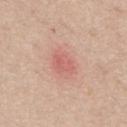Notes:
• workup · total-body-photography surveillance lesion; no biopsy
• tile lighting · white-light
• diameter · ~3.5 mm (longest diameter)
• patient · male, aged approximately 60
• image source · ~15 mm tile from a whole-body skin photo
• location · the mid back